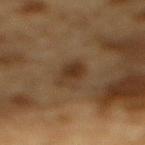• follow-up — no biopsy performed (imaged during a skin exam)